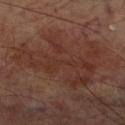Q: Is there a histopathology result?
A: catalogued during a skin exam; not biopsied